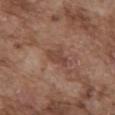  lighting: white-light
  site: abdomen
  lesion_size:
    long_diameter_mm_approx: 3.0
  patient:
    sex: male
    age_approx: 75
  image:
    source: total-body photography crop
    field_of_view_mm: 15